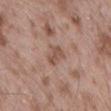patient:
  sex: male
  age_approx: 55
image:
  source: total-body photography crop
  field_of_view_mm: 15
site: back